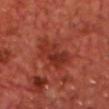• follow-up — no biopsy performed (imaged during a skin exam)
• illumination — cross-polarized illumination
• image — ~15 mm crop, total-body skin-cancer survey
• anatomic site — the chest
• subject — male, aged 68 to 72